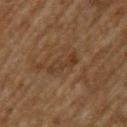Case summary:
- biopsy status · catalogued during a skin exam; not biopsied
- image source · ~15 mm tile from a whole-body skin photo
- body site · the upper back
- patient · male, roughly 65 years of age
- lighting · cross-polarized illumination